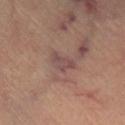Q: Was this lesion biopsied?
A: total-body-photography surveillance lesion; no biopsy
Q: How was this image acquired?
A: 15 mm crop, total-body photography
Q: Patient demographics?
A: female, aged 58–62
Q: Lesion location?
A: the left leg
Q: What did automated image analysis measure?
A: a footprint of about 5 mm², an eccentricity of roughly 0.75, and two-axis asymmetry of about 0.4; a lesion color around L≈47 a*≈19 b*≈20 in CIELAB, about 7 CIELAB-L* units darker than the surrounding skin, and a lesion-to-skin contrast of about 6.5 (normalized; higher = more distinct); a classifier nevus-likeness of about 0/100 and lesion-presence confidence of about 80/100
Q: Lesion size?
A: ≈3 mm